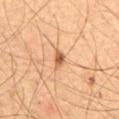Findings:
– location — the mid back
– patient — male, about 65 years old
– lighting — cross-polarized
– image source — ~15 mm crop, total-body skin-cancer survey
– lesion size — ~2.5 mm (longest diameter)